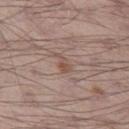notes — imaged on a skin check; not biopsied